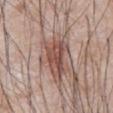Clinical impression: Captured during whole-body skin photography for melanoma surveillance; the lesion was not biopsied.